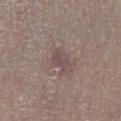Captured during whole-body skin photography for melanoma surveillance; the lesion was not biopsied. Imaged with white-light lighting. The lesion is on the left lower leg. An algorithmic analysis of the crop reported a footprint of about 4.5 mm², a shape eccentricity near 0.75, and two-axis asymmetry of about 0.3. The analysis additionally found border irregularity of about 3 on a 0–10 scale, a color-variation rating of about 2.5/10, and peripheral color asymmetry of about 1. The lesion's longest dimension is about 3 mm. A lesion tile, about 15 mm wide, cut from a 3D total-body photograph. A male subject approximately 55 years of age.